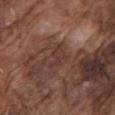Notes:
– body site · the left upper arm
– image · ~15 mm crop, total-body skin-cancer survey
– automated metrics · an area of roughly 4 mm² and a symmetry-axis asymmetry near 0.55; an average lesion color of about L≈36 a*≈20 b*≈22 (CIELAB); a border-irregularity index near 6/10, a color-variation rating of about 1.5/10, and peripheral color asymmetry of about 1; an automated nevus-likeness rating near 0 out of 100 and lesion-presence confidence of about 70/100
– subject · male, in their mid-70s
– size · ≈3 mm
– lighting · white-light